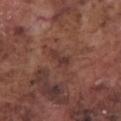Recorded during total-body skin imaging; not selected for excision or biopsy. A male subject in their mid- to late 70s. A roughly 15 mm field-of-view crop from a total-body skin photograph. Longest diameter approximately 3.5 mm. The lesion is on the chest.